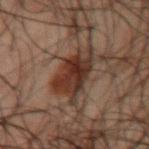biopsy status: total-body-photography surveillance lesion; no biopsy | subject: male, aged 48–52 | imaging modality: 15 mm crop, total-body photography | body site: the left upper arm | diameter: ~6 mm (longest diameter).